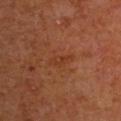{"biopsy_status": "not biopsied; imaged during a skin examination", "automated_metrics": {"area_mm2_approx": 3.0, "eccentricity": 0.9, "shape_asymmetry": 0.4, "cielab_L": 35, "cielab_a": 24, "cielab_b": 33, "vs_skin_darker_L": 5.0, "vs_skin_contrast_norm": 5.0, "border_irregularity_0_10": 4.5, "peripheral_color_asymmetry": 0.0, "nevus_likeness_0_100": 0}, "lesion_size": {"long_diameter_mm_approx": 3.0}, "site": "right upper arm", "image": {"source": "total-body photography crop", "field_of_view_mm": 15}, "patient": {"sex": "male", "age_approx": 65}}